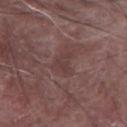{
  "biopsy_status": "not biopsied; imaged during a skin examination",
  "patient": {
    "sex": "male",
    "age_approx": "85+"
  },
  "image": {
    "source": "total-body photography crop",
    "field_of_view_mm": 15
  },
  "lighting": "white-light",
  "site": "right forearm",
  "lesion_size": {
    "long_diameter_mm_approx": 2.5
  }
}